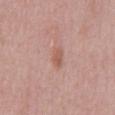The lesion was photographed on a routine skin check and not biopsied; there is no pathology result. A male subject about 50 years old. On the abdomen. Cropped from a total-body skin-imaging series; the visible field is about 15 mm.